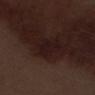notes: imaged on a skin check; not biopsied
site: the left thigh
lighting: white-light
automated metrics: a footprint of about 5 mm² and two-axis asymmetry of about 0.35; a lesion color around L≈15 a*≈15 b*≈14 in CIELAB and a normalized lesion–skin contrast near 7; a border-irregularity rating of about 3.5/10 and peripheral color asymmetry of about 0.5; a classifier nevus-likeness of about 0/100 and a detector confidence of about 75 out of 100 that the crop contains a lesion
subject: male, approximately 70 years of age
lesion size: ~3 mm (longest diameter)
imaging modality: ~15 mm crop, total-body skin-cancer survey